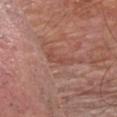Case summary:
– workup — imaged on a skin check; not biopsied
– image source — ~15 mm crop, total-body skin-cancer survey
– site — the chest
– subject — male, aged around 65
– automated metrics — a border-irregularity rating of about 5.5/10, internal color variation of about 0.5 on a 0–10 scale, and a peripheral color-asymmetry measure near 0